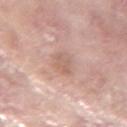{
  "image": {
    "source": "total-body photography crop",
    "field_of_view_mm": 15
  },
  "lighting": "white-light",
  "lesion_size": {
    "long_diameter_mm_approx": 3.0
  },
  "patient": {
    "sex": "female",
    "age_approx": 70
  },
  "site": "left forearm",
  "automated_metrics": {
    "area_mm2_approx": 6.0,
    "vs_skin_darker_L": 7.0,
    "vs_skin_contrast_norm": 5.0,
    "border_irregularity_0_10": 2.0,
    "color_variation_0_10": 2.5,
    "peripheral_color_asymmetry": 1.0
  }
}